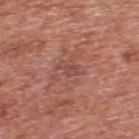Case summary:
- notes · imaged on a skin check; not biopsied
- size · about 2.5 mm
- subject · male, aged around 70
- imaging modality · total-body-photography crop, ~15 mm field of view
- anatomic site · the upper back
- image-analysis metrics · a footprint of about 3 mm², an eccentricity of roughly 0.85, and a shape-asymmetry score of about 0.45 (0 = symmetric); an automated nevus-likeness rating near 0 out of 100